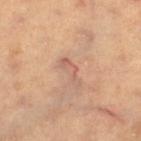Recorded during total-body skin imaging; not selected for excision or biopsy. Measured at roughly 3.5 mm in maximum diameter. The patient is a female approximately 50 years of age. The lesion is on the left thigh. The lesion-visualizer software estimated a lesion area of about 3.5 mm², a shape eccentricity near 0.95, and a symmetry-axis asymmetry near 0.7. The software also gave a border-irregularity rating of about 8.5/10, a color-variation rating of about 0.5/10, and a peripheral color-asymmetry measure near 0. And it measured an automated nevus-likeness rating near 0 out of 100 and a detector confidence of about 85 out of 100 that the crop contains a lesion. A close-up tile cropped from a whole-body skin photograph, about 15 mm across.